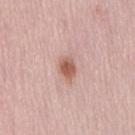| field | value |
|---|---|
| biopsy status | total-body-photography surveillance lesion; no biopsy |